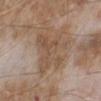notes: no biopsy performed (imaged during a skin exam) | lesion size: ~6 mm (longest diameter) | site: the left lower leg | patient: male, aged approximately 65 | image source: ~15 mm tile from a whole-body skin photo | image-analysis metrics: a lesion area of about 19 mm², an eccentricity of roughly 0.5, and a symmetry-axis asymmetry near 0.35; an average lesion color of about L≈51 a*≈16 b*≈28 (CIELAB) and a lesion-to-skin contrast of about 5.5 (normalized; higher = more distinct); a classifier nevus-likeness of about 0/100 and a lesion-detection confidence of about 100/100.